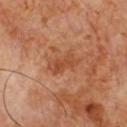Assessment:
Part of a total-body skin-imaging series; this lesion was reviewed on a skin check and was not flagged for biopsy.
Background:
A male patient, approximately 60 years of age. This image is a 15 mm lesion crop taken from a total-body photograph. The lesion's longest dimension is about 3.5 mm. The lesion is on the chest. Imaged with cross-polarized lighting.